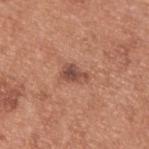The lesion was photographed on a routine skin check and not biopsied; there is no pathology result. From the upper back. This is a white-light tile. A lesion tile, about 15 mm wide, cut from a 3D total-body photograph. Approximately 3 mm at its widest. A male patient, aged approximately 55. An algorithmic analysis of the crop reported roughly 11 lightness units darker than nearby skin and a normalized lesion–skin contrast near 8. And it measured a detector confidence of about 100 out of 100 that the crop contains a lesion.